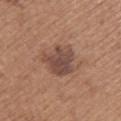Case summary:
• size — ~4.5 mm (longest diameter)
• subject — male, in their mid- to late 60s
• acquisition — ~15 mm tile from a whole-body skin photo
• lighting — white-light
• anatomic site — the back